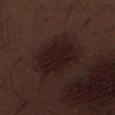Part of a total-body skin-imaging series; this lesion was reviewed on a skin check and was not flagged for biopsy. The total-body-photography lesion software estimated border irregularity of about 2 on a 0–10 scale, a color-variation rating of about 3/10, and peripheral color asymmetry of about 1. A close-up tile cropped from a whole-body skin photograph, about 15 mm across. The lesion's longest dimension is about 6 mm. A male subject, in their 70s. On the right thigh. This is a white-light tile.